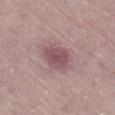Q: How was this image acquired?
A: ~15 mm crop, total-body skin-cancer survey
Q: What are the patient's age and sex?
A: female, approximately 70 years of age
Q: What is the lesion's diameter?
A: about 3.5 mm
Q: How was the tile lit?
A: white-light
Q: Lesion location?
A: the right lower leg